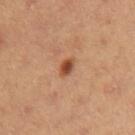Clinical impression:
The lesion was photographed on a routine skin check and not biopsied; there is no pathology result.
Context:
The subject is a female aged approximately 35. A close-up tile cropped from a whole-body skin photograph, about 15 mm across. Approximately 2.5 mm at its widest. Automated tile analysis of the lesion measured a footprint of about 3.5 mm², a shape eccentricity near 0.75, and two-axis asymmetry of about 0.25. It also reported about 13 CIELAB-L* units darker than the surrounding skin and a lesion-to-skin contrast of about 9 (normalized; higher = more distinct). The analysis additionally found a classifier nevus-likeness of about 95/100 and a lesion-detection confidence of about 100/100. From the left upper arm.